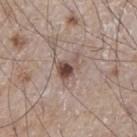Captured during whole-body skin photography for melanoma surveillance; the lesion was not biopsied. This is a white-light tile. A male patient, aged approximately 75. About 4 mm across. A roughly 15 mm field-of-view crop from a total-body skin photograph. The lesion is located on the chest.